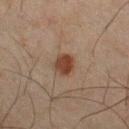Imaged during a routine full-body skin examination; the lesion was not biopsied and no histopathology is available. Cropped from a total-body skin-imaging series; the visible field is about 15 mm. The lesion's longest dimension is about 3 mm. This is a cross-polarized tile. From the right thigh. A male subject, about 45 years old.